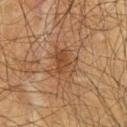Findings:
– acquisition: ~15 mm tile from a whole-body skin photo
– patient: male, in their 60s
– site: the chest